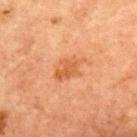• biopsy status: catalogued during a skin exam; not biopsied
• image: ~15 mm tile from a whole-body skin photo
• automated metrics: a lesion area of about 6 mm² and a symmetry-axis asymmetry near 0.25; a mean CIELAB color near L≈48 a*≈24 b*≈36, a lesion–skin lightness drop of about 7, and a lesion-to-skin contrast of about 6 (normalized; higher = more distinct)
• patient: male, aged approximately 65
• lesion diameter: ~4 mm (longest diameter)
• location: the chest